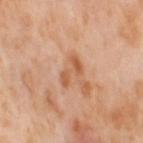  biopsy_status: not biopsied; imaged during a skin examination
  site: right thigh
  patient:
    sex: female
    age_approx: 55
  image:
    source: total-body photography crop
    field_of_view_mm: 15